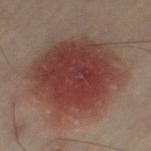Q: Is there a histopathology result?
A: no biopsy performed (imaged during a skin exam)
Q: What kind of image is this?
A: ~15 mm crop, total-body skin-cancer survey
Q: What did automated image analysis measure?
A: an average lesion color of about L≈31 a*≈19 b*≈19 (CIELAB), roughly 10 lightness units darker than nearby skin, and a lesion-to-skin contrast of about 10 (normalized; higher = more distinct); a classifier nevus-likeness of about 100/100 and lesion-presence confidence of about 100/100
Q: What lighting was used for the tile?
A: cross-polarized
Q: Who is the patient?
A: male, aged around 50
Q: Where on the body is the lesion?
A: the left thigh
Q: How large is the lesion?
A: ~10.5 mm (longest diameter)